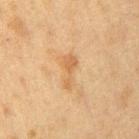{"biopsy_status": "not biopsied; imaged during a skin examination", "site": "arm", "lesion_size": {"long_diameter_mm_approx": 4.0}, "patient": {"sex": "female", "age_approx": 40}, "automated_metrics": {"eccentricity": 0.9, "nevus_likeness_0_100": 0, "lesion_detection_confidence_0_100": 100}, "image": {"source": "total-body photography crop", "field_of_view_mm": 15}, "lighting": "cross-polarized"}